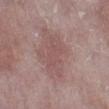notes — total-body-photography surveillance lesion; no biopsy
site — the left lower leg
TBP lesion metrics — an eccentricity of roughly 0.9; about 6 CIELAB-L* units darker than the surrounding skin and a normalized lesion–skin contrast near 4.5; lesion-presence confidence of about 100/100
subject — female, aged 63 to 67
acquisition — total-body-photography crop, ~15 mm field of view